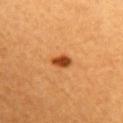{"site": "chest", "lesion_size": {"long_diameter_mm_approx": 2.5}, "automated_metrics": {"cielab_L": 45, "cielab_a": 29, "cielab_b": 43, "vs_skin_darker_L": 15.0, "vs_skin_contrast_norm": 10.5, "nevus_likeness_0_100": 100}, "image": {"source": "total-body photography crop", "field_of_view_mm": 15}, "lighting": "cross-polarized", "patient": {"sex": "female", "age_approx": 30}}